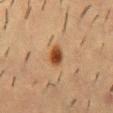This lesion was catalogued during total-body skin photography and was not selected for biopsy.
Approximately 2.5 mm at its widest.
The patient is a male aged 53 to 57.
The lesion is on the mid back.
A 15 mm close-up extracted from a 3D total-body photography capture.